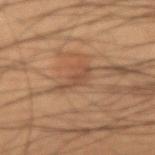follow-up=total-body-photography surveillance lesion; no biopsy | patient=male, aged 48 to 52 | automated lesion analysis=an area of roughly 5 mm²; border irregularity of about 5 on a 0–10 scale, internal color variation of about 1 on a 0–10 scale, and a peripheral color-asymmetry measure near 0.5; a classifier nevus-likeness of about 0/100 and a lesion-detection confidence of about 75/100 | anatomic site=the right forearm | image source=15 mm crop, total-body photography.